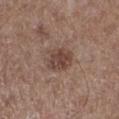* notes: no biopsy performed (imaged during a skin exam)
* patient: male, aged around 60
* image: total-body-photography crop, ~15 mm field of view
* lighting: white-light illumination
* site: the left lower leg
* TBP lesion metrics: an outline eccentricity of about 0.55 (0 = round, 1 = elongated) and a shape-asymmetry score of about 0.2 (0 = symmetric); an average lesion color of about L≈43 a*≈17 b*≈23 (CIELAB) and a normalized lesion–skin contrast near 7.5; a border-irregularity index near 2/10 and a color-variation rating of about 3.5/10; an automated nevus-likeness rating near 45 out of 100 and lesion-presence confidence of about 100/100